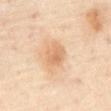Imaged during a routine full-body skin examination; the lesion was not biopsied and no histopathology is available. A male patient aged 63–67. This image is a 15 mm lesion crop taken from a total-body photograph. An algorithmic analysis of the crop reported about 9 CIELAB-L* units darker than the surrounding skin and a normalized border contrast of about 6. The lesion is located on the abdomen.